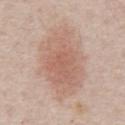Clinical impression:
Captured during whole-body skin photography for melanoma surveillance; the lesion was not biopsied.
Image and clinical context:
The lesion's longest dimension is about 9.5 mm. The patient is a male roughly 60 years of age. A 15 mm close-up extracted from a 3D total-body photography capture. Automated image analysis of the tile measured a lesion color around L≈62 a*≈19 b*≈27 in CIELAB, roughly 9 lightness units darker than nearby skin, and a normalized border contrast of about 6. It also reported a detector confidence of about 100 out of 100 that the crop contains a lesion. On the abdomen.